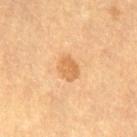Q: Is there a histopathology result?
A: total-body-photography surveillance lesion; no biopsy
Q: Where on the body is the lesion?
A: the leg
Q: What kind of image is this?
A: 15 mm crop, total-body photography
Q: Patient demographics?
A: female, aged approximately 70
Q: Illumination type?
A: cross-polarized
Q: What is the lesion's diameter?
A: about 2.5 mm